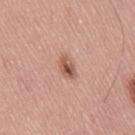Findings:
- notes: catalogued during a skin exam; not biopsied
- site: the leg
- illumination: white-light
- imaging modality: ~15 mm crop, total-body skin-cancer survey
- patient: male, about 55 years old